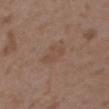No biopsy was performed on this lesion — it was imaged during a full skin examination and was not determined to be concerning. On the upper back. A female subject, roughly 35 years of age. About 3.5 mm across. A 15 mm crop from a total-body photograph taken for skin-cancer surveillance.